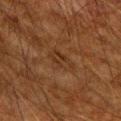notes: catalogued during a skin exam; not biopsied
TBP lesion metrics: an area of roughly 6 mm², an eccentricity of roughly 0.85, and a shape-asymmetry score of about 0.25 (0 = symmetric); border irregularity of about 3 on a 0–10 scale, a within-lesion color-variation index near 3/10, and a peripheral color-asymmetry measure near 1
site: the right upper arm
illumination: cross-polarized
subject: male, in their mid- to late 60s
image source: ~15 mm tile from a whole-body skin photo
lesion diameter: ≈4 mm